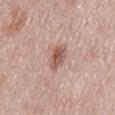Assessment:
No biopsy was performed on this lesion — it was imaged during a full skin examination and was not determined to be concerning.
Background:
The lesion is located on the mid back. The patient is a male aged 53 to 57. Longest diameter approximately 4 mm. Automated image analysis of the tile measured internal color variation of about 3.5 on a 0–10 scale. The analysis additionally found a nevus-likeness score of about 95/100 and a lesion-detection confidence of about 100/100. A region of skin cropped from a whole-body photographic capture, roughly 15 mm wide. Captured under white-light illumination.